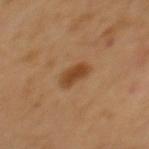Q: Was a biopsy performed?
A: total-body-photography surveillance lesion; no biopsy
Q: What is the anatomic site?
A: the upper back
Q: Automated lesion metrics?
A: a footprint of about 5 mm², an outline eccentricity of about 0.8 (0 = round, 1 = elongated), and two-axis asymmetry of about 0.2; a border-irregularity rating of about 2/10, a within-lesion color-variation index near 2/10, and peripheral color asymmetry of about 0.5
Q: How was this image acquired?
A: ~15 mm tile from a whole-body skin photo
Q: How was the tile lit?
A: cross-polarized illumination
Q: Who is the patient?
A: male, in their mid- to late 60s